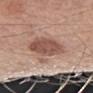Clinical impression: Recorded during total-body skin imaging; not selected for excision or biopsy. Context: The recorded lesion diameter is about 5 mm. A 15 mm close-up tile from a total-body photography series done for melanoma screening. A male patient, aged 58–62. The lesion is on the left forearm. Imaged with white-light lighting.